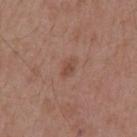notes — total-body-photography surveillance lesion; no biopsy | site — the back | imaging modality — ~15 mm tile from a whole-body skin photo | patient — male, aged 53–57.